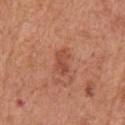| feature | finding |
|---|---|
| lighting | white-light |
| image source | ~15 mm tile from a whole-body skin photo |
| automated lesion analysis | a mean CIELAB color near L≈49 a*≈28 b*≈32, roughly 9 lightness units darker than nearby skin, and a normalized border contrast of about 6; a nevus-likeness score of about 10/100 and lesion-presence confidence of about 100/100 |
| location | the chest |
| patient | male, in their mid- to late 60s |
| lesion size | ~3 mm (longest diameter) |